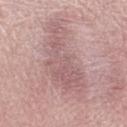* workup: no biopsy performed (imaged during a skin exam)
* anatomic site: the leg
* subject: female, aged 63–67
* acquisition: 15 mm crop, total-body photography
* size: about 10 mm
* automated lesion analysis: a footprint of about 23 mm², an eccentricity of roughly 0.95, and a shape-asymmetry score of about 0.45 (0 = symmetric); an average lesion color of about L≈58 a*≈21 b*≈19 (CIELAB), a lesion–skin lightness drop of about 8, and a lesion-to-skin contrast of about 5.5 (normalized; higher = more distinct); an automated nevus-likeness rating near 0 out of 100 and a detector confidence of about 50 out of 100 that the crop contains a lesion